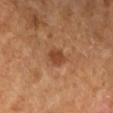Recorded during total-body skin imaging; not selected for excision or biopsy. The patient is aged 68 to 72. A 15 mm crop from a total-body photograph taken for skin-cancer surveillance. On the left lower leg.